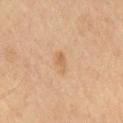The subject is a male aged 63 to 67. A region of skin cropped from a whole-body photographic capture, roughly 15 mm wide. Located on the chest.